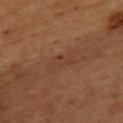notes — no biopsy performed (imaged during a skin exam) | diameter — ≈4 mm | image-analysis metrics — an area of roughly 5.5 mm², an eccentricity of roughly 0.9, and a shape-asymmetry score of about 0.35 (0 = symmetric); a lesion-detection confidence of about 95/100 | subject — male, aged 58 to 62 | site — the chest | image — ~15 mm crop, total-body skin-cancer survey.